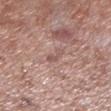follow-up = no biopsy performed (imaged during a skin exam) | diameter = ~3 mm (longest diameter) | anatomic site = the left lower leg | image = ~15 mm tile from a whole-body skin photo | patient = male, aged around 55.